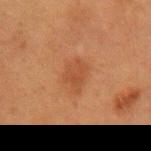Notes:
* notes — catalogued during a skin exam; not biopsied
* lesion diameter — about 3.5 mm
* subject — female, aged 38 to 42
* automated lesion analysis — an area of roughly 6.5 mm², a shape eccentricity near 0.7, and a symmetry-axis asymmetry near 0.3; border irregularity of about 3 on a 0–10 scale, internal color variation of about 2 on a 0–10 scale, and radial color variation of about 0.5
* imaging modality — total-body-photography crop, ~15 mm field of view
* body site — the left upper arm